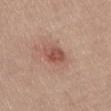{
  "biopsy_status": "not biopsied; imaged during a skin examination",
  "lesion_size": {
    "long_diameter_mm_approx": 3.0
  },
  "patient": {
    "sex": "male",
    "age_approx": 30
  },
  "image": {
    "source": "total-body photography crop",
    "field_of_view_mm": 15
  },
  "lighting": "white-light",
  "site": "abdomen",
  "automated_metrics": {
    "cielab_L": 52,
    "cielab_a": 24,
    "cielab_b": 27,
    "vs_skin_darker_L": 10.0,
    "border_irregularity_0_10": 1.5,
    "color_variation_0_10": 3.5,
    "nevus_likeness_0_100": 80,
    "lesion_detection_confidence_0_100": 100
  }
}